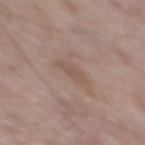The lesion was tiled from a total-body skin photograph and was not biopsied. The tile uses white-light illumination. The lesion is on the upper back. A male subject aged around 65. The lesion-visualizer software estimated a lesion area of about 4 mm², an outline eccentricity of about 0.9 (0 = round, 1 = elongated), and a shape-asymmetry score of about 0.3 (0 = symmetric). The software also gave border irregularity of about 3.5 on a 0–10 scale. A 15 mm close-up extracted from a 3D total-body photography capture. About 3.5 mm across.